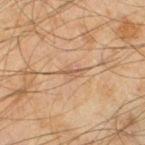Q: Lesion location?
A: the left thigh
Q: What kind of image is this?
A: ~15 mm crop, total-body skin-cancer survey
Q: What are the patient's age and sex?
A: male, roughly 45 years of age